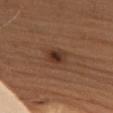Captured during whole-body skin photography for melanoma surveillance; the lesion was not biopsied.
About 3 mm across.
The lesion-visualizer software estimated a lesion area of about 5 mm², a shape eccentricity near 0.8, and a symmetry-axis asymmetry near 0.15. It also reported an average lesion color of about L≈34 a*≈19 b*≈27 (CIELAB) and a normalized lesion–skin contrast near 9. The analysis additionally found a border-irregularity rating of about 1.5/10 and a peripheral color-asymmetry measure near 2. The analysis additionally found a nevus-likeness score of about 95/100 and a lesion-detection confidence of about 100/100.
A roughly 15 mm field-of-view crop from a total-body skin photograph.
The subject is a male roughly 70 years of age.
Imaged with white-light lighting.
Located on the chest.